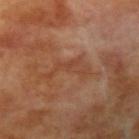This lesion was catalogued during total-body skin photography and was not selected for biopsy. The total-body-photography lesion software estimated a mean CIELAB color near L≈43 a*≈23 b*≈31, roughly 6 lightness units darker than nearby skin, and a lesion-to-skin contrast of about 5 (normalized; higher = more distinct). The software also gave border irregularity of about 9.5 on a 0–10 scale, a within-lesion color-variation index near 1.5/10, and peripheral color asymmetry of about 0.5. A male patient, aged 68 to 72. This image is a 15 mm lesion crop taken from a total-body photograph. Imaged with cross-polarized lighting. On the arm.